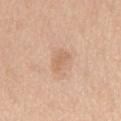This lesion was catalogued during total-body skin photography and was not selected for biopsy. The total-body-photography lesion software estimated an outline eccentricity of about 0.85 (0 = round, 1 = elongated). It also reported an average lesion color of about L≈64 a*≈19 b*≈32 (CIELAB), roughly 7 lightness units darker than nearby skin, and a normalized lesion–skin contrast near 5. The analysis additionally found border irregularity of about 3.5 on a 0–10 scale, internal color variation of about 1 on a 0–10 scale, and radial color variation of about 0.5. This image is a 15 mm lesion crop taken from a total-body photograph. About 3 mm across. A male subject in their 60s. The lesion is located on the front of the torso.